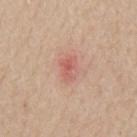Impression: The lesion was tiled from a total-body skin photograph and was not biopsied. Context: The lesion's longest dimension is about 3 mm. The tile uses white-light illumination. A male subject aged 58 to 62. A 15 mm close-up extracted from a 3D total-body photography capture. The lesion is on the mid back.